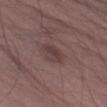Clinical impression:
This lesion was catalogued during total-body skin photography and was not selected for biopsy.
Clinical summary:
A 15 mm close-up tile from a total-body photography series done for melanoma screening. This is a white-light tile. Longest diameter approximately 3.5 mm. The lesion-visualizer software estimated a lesion area of about 6 mm², a shape eccentricity near 0.8, and a shape-asymmetry score of about 0.2 (0 = symmetric). The analysis additionally found an average lesion color of about L≈39 a*≈17 b*≈17 (CIELAB), a lesion–skin lightness drop of about 7, and a normalized border contrast of about 6. The software also gave internal color variation of about 3 on a 0–10 scale and radial color variation of about 1. The analysis additionally found an automated nevus-likeness rating near 0 out of 100 and lesion-presence confidence of about 100/100. A male patient, roughly 50 years of age. Located on the left upper arm.